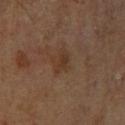Notes:
* workup: total-body-photography surveillance lesion; no biopsy
* anatomic site: the right leg
* size: about 3 mm
* patient: male, aged around 60
* acquisition: ~15 mm tile from a whole-body skin photo
* illumination: cross-polarized illumination
* image-analysis metrics: a footprint of about 3.5 mm², an outline eccentricity of about 0.9 (0 = round, 1 = elongated), and a symmetry-axis asymmetry near 0.4; a mean CIELAB color near L≈32 a*≈16 b*≈26, roughly 6 lightness units darker than nearby skin, and a lesion-to-skin contrast of about 6.5 (normalized; higher = more distinct)